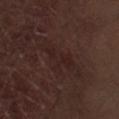biopsy_status: not biopsied; imaged during a skin examination
automated_metrics:
  vs_skin_darker_L: 4.0
  vs_skin_contrast_norm: 5.0
  nevus_likeness_0_100: 0
  lesion_detection_confidence_0_100: 80
lesion_size:
  long_diameter_mm_approx: 5.0
patient:
  sex: male
  age_approx: 70
image:
  source: total-body photography crop
  field_of_view_mm: 15
lighting: white-light
site: right thigh